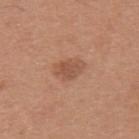Case summary:
– anatomic site: the upper back
– subject: male, aged around 30
– lesion size: ~3.5 mm (longest diameter)
– image: 15 mm crop, total-body photography
– automated lesion analysis: an area of roughly 6.5 mm², a shape eccentricity near 0.75, and two-axis asymmetry of about 0.2; internal color variation of about 2 on a 0–10 scale and peripheral color asymmetry of about 0.5; an automated nevus-likeness rating near 50 out of 100 and lesion-presence confidence of about 100/100